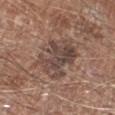Q: Is there a histopathology result?
A: no biopsy performed (imaged during a skin exam)
Q: How was this image acquired?
A: 15 mm crop, total-body photography
Q: Who is the patient?
A: male, roughly 80 years of age
Q: Lesion location?
A: the right lower leg
Q: Automated lesion metrics?
A: a border-irregularity index near 5.5/10 and peripheral color asymmetry of about 2; an automated nevus-likeness rating near 5 out of 100 and a detector confidence of about 100 out of 100 that the crop contains a lesion